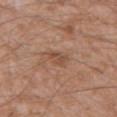| feature | finding |
|---|---|
| biopsy status | imaged on a skin check; not biopsied |
| lesion size | about 2.5 mm |
| body site | the mid back |
| image | 15 mm crop, total-body photography |
| subject | male, in their 60s |
| illumination | white-light |